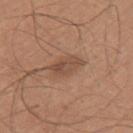| feature | finding |
|---|---|
| notes | total-body-photography surveillance lesion; no biopsy |
| patient | male, about 30 years old |
| anatomic site | the left upper arm |
| lighting | white-light illumination |
| image source | ~15 mm crop, total-body skin-cancer survey |
| image-analysis metrics | an average lesion color of about L≈49 a*≈19 b*≈29 (CIELAB) |
| diameter | ~4.5 mm (longest diameter) |